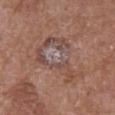The total-body-photography lesion software estimated an outline eccentricity of about 0.75 (0 = round, 1 = elongated) and two-axis asymmetry of about 0.65. The analysis additionally found an average lesion color of about L≈49 a*≈17 b*≈22 (CIELAB), a lesion–skin lightness drop of about 5, and a normalized border contrast of about 4.5. The analysis additionally found a classifier nevus-likeness of about 0/100 and lesion-presence confidence of about 95/100. From the chest. A female subject roughly 70 years of age. A 15 mm crop from a total-body photograph taken for skin-cancer surveillance.